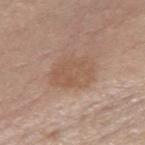Case summary:
– biopsy status: no biopsy performed (imaged during a skin exam)
– image: ~15 mm crop, total-body skin-cancer survey
– tile lighting: white-light
– TBP lesion metrics: a symmetry-axis asymmetry near 0.15; a border-irregularity rating of about 2/10, a within-lesion color-variation index near 2.5/10, and peripheral color asymmetry of about 1; a nevus-likeness score of about 0/100 and lesion-presence confidence of about 100/100
– patient: female, roughly 65 years of age
– anatomic site: the right forearm